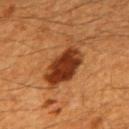Captured during whole-body skin photography for melanoma surveillance; the lesion was not biopsied. The lesion-visualizer software estimated a mean CIELAB color near L≈36 a*≈25 b*≈35, roughly 15 lightness units darker than nearby skin, and a lesion-to-skin contrast of about 12 (normalized; higher = more distinct). It also reported border irregularity of about 4.5 on a 0–10 scale and internal color variation of about 6.5 on a 0–10 scale. And it measured lesion-presence confidence of about 100/100. Located on the mid back. This is a cross-polarized tile. The patient is a male in their 60s. The recorded lesion diameter is about 7.5 mm. A 15 mm close-up extracted from a 3D total-body photography capture.